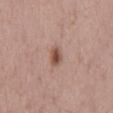Recorded during total-body skin imaging; not selected for excision or biopsy.
The lesion is on the mid back.
This is a white-light tile.
The patient is a male in their 50s.
A 15 mm close-up extracted from a 3D total-body photography capture.
The recorded lesion diameter is about 2.5 mm.
Automated tile analysis of the lesion measured an area of roughly 4 mm², an outline eccentricity of about 0.8 (0 = round, 1 = elongated), and a symmetry-axis asymmetry near 0.2. The analysis additionally found a lesion color around L≈51 a*≈21 b*≈26 in CIELAB, roughly 12 lightness units darker than nearby skin, and a lesion-to-skin contrast of about 8.5 (normalized; higher = more distinct). The analysis additionally found a border-irregularity index near 1.5/10 and a within-lesion color-variation index near 5.5/10.